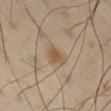Q: Was this lesion biopsied?
A: no biopsy performed (imaged during a skin exam)
Q: Who is the patient?
A: male, aged around 55
Q: How large is the lesion?
A: ≈2.5 mm
Q: Illumination type?
A: cross-polarized
Q: What did automated image analysis measure?
A: a normalized lesion–skin contrast near 7; border irregularity of about 2.5 on a 0–10 scale, internal color variation of about 2.5 on a 0–10 scale, and a peripheral color-asymmetry measure near 0.5
Q: What is the imaging modality?
A: total-body-photography crop, ~15 mm field of view
Q: Lesion location?
A: the right thigh